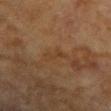No biopsy was performed on this lesion — it was imaged during a full skin examination and was not determined to be concerning.
The total-body-photography lesion software estimated a footprint of about 3.5 mm², a shape eccentricity near 0.95, and two-axis asymmetry of about 0.45. The analysis additionally found roughly 4 lightness units darker than nearby skin and a lesion-to-skin contrast of about 4.5 (normalized; higher = more distinct). The software also gave a border-irregularity rating of about 5.5/10 and a color-variation rating of about 0/10.
The lesion is on the right forearm.
A region of skin cropped from a whole-body photographic capture, roughly 15 mm wide.
A female patient, approximately 80 years of age.
Captured under cross-polarized illumination.